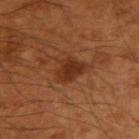{"biopsy_status": "not biopsied; imaged during a skin examination", "patient": {"sex": "male", "age_approx": 50}, "image": {"source": "total-body photography crop", "field_of_view_mm": 15}, "automated_metrics": {"area_mm2_approx": 7.0, "eccentricity": 0.5, "shape_asymmetry": 0.3, "nevus_likeness_0_100": 70, "lesion_detection_confidence_0_100": 100}, "lesion_size": {"long_diameter_mm_approx": 3.5}, "site": "left arm", "lighting": "cross-polarized"}